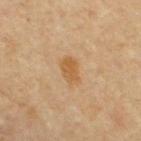Impression: Part of a total-body skin-imaging series; this lesion was reviewed on a skin check and was not flagged for biopsy. Clinical summary: The total-body-photography lesion software estimated an automated nevus-likeness rating near 80 out of 100 and a lesion-detection confidence of about 100/100. The patient is a male about 65 years old. From the chest. The recorded lesion diameter is about 3 mm. Cropped from a whole-body photographic skin survey; the tile spans about 15 mm.